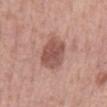Findings:
- biopsy status — imaged on a skin check; not biopsied
- patient — male, approximately 55 years of age
- lesion diameter — ≈5.5 mm
- image source — total-body-photography crop, ~15 mm field of view
- image-analysis metrics — a peripheral color-asymmetry measure near 1
- site — the back
- illumination — white-light illumination